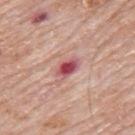Findings:
- biopsy status · no biopsy performed (imaged during a skin exam)